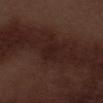notes: catalogued during a skin exam; not biopsied | TBP lesion metrics: a footprint of about 5 mm², a shape eccentricity near 0.4, and a symmetry-axis asymmetry near 0.25; a classifier nevus-likeness of about 0/100 and a detector confidence of about 95 out of 100 that the crop contains a lesion | imaging modality: ~15 mm tile from a whole-body skin photo | illumination: white-light illumination | anatomic site: the left thigh | diameter: ≈2.5 mm | patient: male, in their 70s.